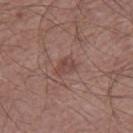Clinical impression:
Part of a total-body skin-imaging series; this lesion was reviewed on a skin check and was not flagged for biopsy.